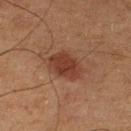<tbp_lesion>
  <site>left lower leg</site>
  <lighting>cross-polarized</lighting>
  <patient>
    <sex>male</sex>
    <age_approx>75</age_approx>
  </patient>
  <image>
    <source>total-body photography crop</source>
    <field_of_view_mm>15</field_of_view_mm>
  </image>
  <lesion_size>
    <long_diameter_mm_approx>4.0</long_diameter_mm_approx>
  </lesion_size>
</tbp_lesion>